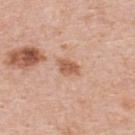Findings:
- notes: no biopsy performed (imaged during a skin exam)
- acquisition: ~15 mm crop, total-body skin-cancer survey
- illumination: white-light
- location: the upper back
- patient: male, roughly 60 years of age